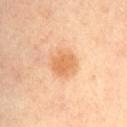biopsy status = total-body-photography surveillance lesion; no biopsy | image-analysis metrics = a peripheral color-asymmetry measure near 1 | acquisition = ~15 mm tile from a whole-body skin photo | diameter = about 3.5 mm | body site = the lower back | patient = male, aged 58–62.